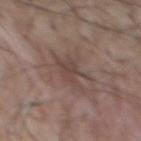lesion size: ~4.5 mm (longest diameter)
illumination: white-light illumination
imaging modality: ~15 mm crop, total-body skin-cancer survey
anatomic site: the chest
subject: male, roughly 75 years of age